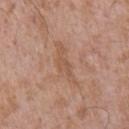location: the right upper arm
image: ~15 mm crop, total-body skin-cancer survey
illumination: white-light illumination
size: ≈6 mm
patient: male, approximately 50 years of age